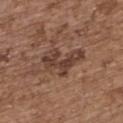notes: imaged on a skin check; not biopsied | diameter: ≈5.5 mm | anatomic site: the upper back | imaging modality: ~15 mm tile from a whole-body skin photo | patient: female, aged 63–67 | tile lighting: white-light illumination.